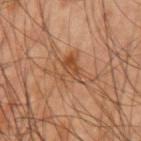| feature | finding |
|---|---|
| biopsy status | total-body-photography surveillance lesion; no biopsy |
| anatomic site | the left upper arm |
| patient | male, approximately 45 years of age |
| tile lighting | cross-polarized illumination |
| acquisition | ~15 mm tile from a whole-body skin photo |
| diameter | ≈5 mm |